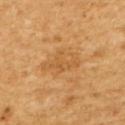Imaged during a routine full-body skin examination; the lesion was not biopsied and no histopathology is available.
This is a cross-polarized tile.
A male subject about 60 years old.
The lesion's longest dimension is about 4.5 mm.
A 15 mm close-up extracted from a 3D total-body photography capture.
The lesion is located on the upper back.